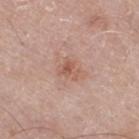{
  "biopsy_status": "not biopsied; imaged during a skin examination",
  "patient": {
    "sex": "male",
    "age_approx": 80
  },
  "site": "right thigh",
  "image": {
    "source": "total-body photography crop",
    "field_of_view_mm": 15
  }
}